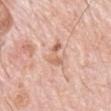| field | value |
|---|---|
| biopsy status | catalogued during a skin exam; not biopsied |
| automated metrics | a lesion area of about 5 mm², a shape eccentricity near 0.85, and a symmetry-axis asymmetry near 0.5 |
| size | ≈3.5 mm |
| illumination | white-light illumination |
| patient | male, aged 78 to 82 |
| anatomic site | the mid back |
| acquisition | total-body-photography crop, ~15 mm field of view |